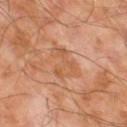{
  "image": {
    "source": "total-body photography crop",
    "field_of_view_mm": 15
  },
  "patient": {
    "sex": "male",
    "age_approx": 65
  },
  "lighting": "cross-polarized",
  "lesion_size": {
    "long_diameter_mm_approx": 5.0
  }
}